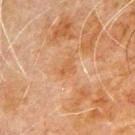No biopsy was performed on this lesion — it was imaged during a full skin examination and was not determined to be concerning. A male subject, in their 80s. The recorded lesion diameter is about 2.5 mm. Cropped from a total-body skin-imaging series; the visible field is about 15 mm. Located on the left upper arm.